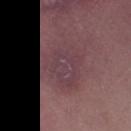Impression:
This lesion was catalogued during total-body skin photography and was not selected for biopsy.
Acquisition and patient details:
Longest diameter approximately 5.5 mm. A 15 mm close-up extracted from a 3D total-body photography capture. The lesion is on the left thigh. A female subject aged approximately 55.